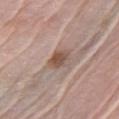Findings:
– workup — catalogued during a skin exam; not biopsied
– lighting — white-light
– site — the left upper arm
– subject — female, aged 63 to 67
– diameter — ~2.5 mm (longest diameter)
– image source — 15 mm crop, total-body photography